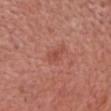The lesion was photographed on a routine skin check and not biopsied; there is no pathology result. A male patient in their 80s. A roughly 15 mm field-of-view crop from a total-body skin photograph. From the chest.